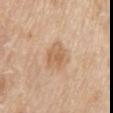Clinical impression:
Captured during whole-body skin photography for melanoma surveillance; the lesion was not biopsied.
Acquisition and patient details:
A 15 mm close-up tile from a total-body photography series done for melanoma screening. Automated tile analysis of the lesion measured an average lesion color of about L≈62 a*≈19 b*≈35 (CIELAB), a lesion–skin lightness drop of about 9, and a lesion-to-skin contrast of about 6 (normalized; higher = more distinct). A female patient aged 63 to 67. Captured under white-light illumination. The lesion is on the arm.